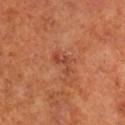illumination = cross-polarized illumination | diameter = ~3 mm (longest diameter) | image = ~15 mm crop, total-body skin-cancer survey | body site = the right lower leg | subject = male, approximately 70 years of age.